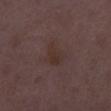Recorded during total-body skin imaging; not selected for excision or biopsy. Cropped from a total-body skin-imaging series; the visible field is about 15 mm. The total-body-photography lesion software estimated a shape eccentricity near 0.5 and a symmetry-axis asymmetry near 0.4. The analysis additionally found an average lesion color of about L≈31 a*≈15 b*≈18 (CIELAB), roughly 4 lightness units darker than nearby skin, and a lesion-to-skin contrast of about 5.5 (normalized; higher = more distinct). The software also gave a nevus-likeness score of about 0/100 and a lesion-detection confidence of about 100/100. The lesion is located on the right thigh. The tile uses white-light illumination. A female subject, in their 30s.